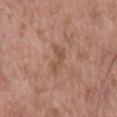No biopsy was performed on this lesion — it was imaged during a full skin examination and was not determined to be concerning. From the mid back. The subject is a male about 55 years old. A lesion tile, about 15 mm wide, cut from a 3D total-body photograph. Longest diameter approximately 3.5 mm.